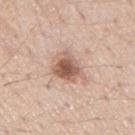- workup — imaged on a skin check; not biopsied
- size — about 4 mm
- image — ~15 mm tile from a whole-body skin photo
- location — the back
- patient — male, roughly 55 years of age
- tile lighting — white-light